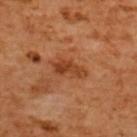Impression: Captured during whole-body skin photography for melanoma surveillance; the lesion was not biopsied. Acquisition and patient details: A lesion tile, about 15 mm wide, cut from a 3D total-body photograph. The lesion-visualizer software estimated a nevus-likeness score of about 0/100 and lesion-presence confidence of about 100/100. A female patient aged 53 to 57. On the upper back. About 4 mm across.